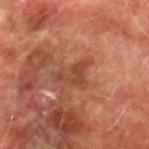acquisition: total-body-photography crop, ~15 mm field of view | subject: male, aged 73 to 77 | body site: the right thigh | automated metrics: a shape-asymmetry score of about 0.7 (0 = symmetric); a classifier nevus-likeness of about 0/100 and a lesion-detection confidence of about 95/100 | size: ≈4 mm | illumination: cross-polarized.